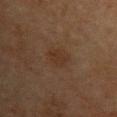<lesion>
<lesion_size>
  <long_diameter_mm_approx>3.0</long_diameter_mm_approx>
</lesion_size>
<patient>
  <sex>female</sex>
  <age_approx>70</age_approx>
</patient>
<site>chest</site>
<lighting>cross-polarized</lighting>
<image>
  <source>total-body photography crop</source>
  <field_of_view_mm>15</field_of_view_mm>
</image>
</lesion>